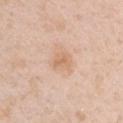Recorded during total-body skin imaging; not selected for excision or biopsy.
The lesion is located on the left upper arm.
This is a white-light tile.
Cropped from a total-body skin-imaging series; the visible field is about 15 mm.
A male patient aged 48–52.